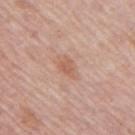Assessment: No biopsy was performed on this lesion — it was imaged during a full skin examination and was not determined to be concerning. Context: The lesion is on the right upper arm. The patient is a female approximately 60 years of age. Measured at roughly 2.5 mm in maximum diameter. A 15 mm close-up extracted from a 3D total-body photography capture. An algorithmic analysis of the crop reported a footprint of about 3.5 mm², a shape eccentricity near 0.7, and a symmetry-axis asymmetry near 0.35. The analysis additionally found an average lesion color of about L≈59 a*≈22 b*≈30 (CIELAB) and a normalized border contrast of about 6.